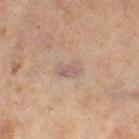{"biopsy_status": "not biopsied; imaged during a skin examination", "patient": {"sex": "female", "age_approx": 60}, "automated_metrics": {"area_mm2_approx": 5.0, "shape_asymmetry": 0.2, "cielab_L": 51, "cielab_a": 14, "cielab_b": 20, "vs_skin_darker_L": 7.0, "vs_skin_contrast_norm": 6.0, "color_variation_0_10": 2.5, "peripheral_color_asymmetry": 1.0, "nevus_likeness_0_100": 0, "lesion_detection_confidence_0_100": 90}, "site": "left thigh", "image": {"source": "total-body photography crop", "field_of_view_mm": 15}}